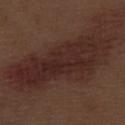Q: Is there a histopathology result?
A: no biopsy performed (imaged during a skin exam)
Q: What lighting was used for the tile?
A: white-light
Q: Where on the body is the lesion?
A: the right thigh
Q: Automated lesion metrics?
A: a mean CIELAB color near L≈26 a*≈18 b*≈20 and about 8 CIELAB-L* units darker than the surrounding skin; a classifier nevus-likeness of about 0/100
Q: Who is the patient?
A: male, aged 68–72
Q: What kind of image is this?
A: ~15 mm tile from a whole-body skin photo